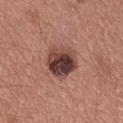{
  "lesion_size": {
    "long_diameter_mm_approx": 4.5
  },
  "site": "chest",
  "image": {
    "source": "total-body photography crop",
    "field_of_view_mm": 15
  },
  "lighting": "white-light",
  "automated_metrics": {
    "cielab_L": 41,
    "cielab_a": 21,
    "cielab_b": 23,
    "vs_skin_darker_L": 16.0,
    "vs_skin_contrast_norm": 12.0,
    "color_variation_0_10": 9.0,
    "peripheral_color_asymmetry": 2.5,
    "lesion_detection_confidence_0_100": 100
  },
  "patient": {
    "sex": "male",
    "age_approx": 35
  }
}